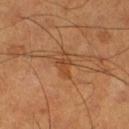Assessment: No biopsy was performed on this lesion — it was imaged during a full skin examination and was not determined to be concerning. Acquisition and patient details: On the left lower leg. This is a cross-polarized tile. The recorded lesion diameter is about 3 mm. A close-up tile cropped from a whole-body skin photograph, about 15 mm across. A male subject, in their mid-50s.